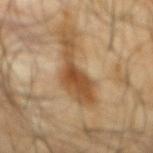follow-up — no biopsy performed (imaged during a skin exam)
anatomic site — the back
acquisition — ~15 mm crop, total-body skin-cancer survey
lesion diameter — ≈7 mm
image-analysis metrics — a lesion area of about 10 mm² and a shape eccentricity near 0.95; a color-variation rating of about 3/10 and peripheral color asymmetry of about 0.5
patient — male, in their mid- to late 60s